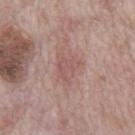| feature | finding |
|---|---|
| location | the abdomen |
| lighting | white-light |
| subject | male, roughly 70 years of age |
| image | ~15 mm crop, total-body skin-cancer survey |
| automated metrics | a footprint of about 6.5 mm², an eccentricity of roughly 0.6, and a shape-asymmetry score of about 0.4 (0 = symmetric) |
| lesion size | ~3.5 mm (longest diameter) |